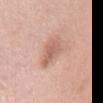follow-up: imaged on a skin check; not biopsied
image: total-body-photography crop, ~15 mm field of view
image-analysis metrics: an automated nevus-likeness rating near 20 out of 100 and a detector confidence of about 100 out of 100 that the crop contains a lesion
patient: female, about 65 years old
illumination: white-light
site: the chest